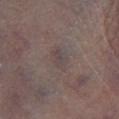Part of a total-body skin-imaging series; this lesion was reviewed on a skin check and was not flagged for biopsy.